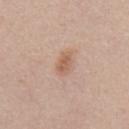| key | value |
|---|---|
| subject | male, aged 53 to 57 |
| illumination | white-light |
| lesion size | about 3 mm |
| TBP lesion metrics | a symmetry-axis asymmetry near 0.2; a mean CIELAB color near L≈59 a*≈19 b*≈30, about 9 CIELAB-L* units darker than the surrounding skin, and a normalized lesion–skin contrast near 6.5; a border-irregularity index near 2/10, a color-variation rating of about 2/10, and radial color variation of about 1 |
| location | the front of the torso |
| imaging modality | ~15 mm tile from a whole-body skin photo |